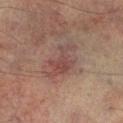Captured during whole-body skin photography for melanoma surveillance; the lesion was not biopsied. This image is a 15 mm lesion crop taken from a total-body photograph. This is a cross-polarized tile. Approximately 5 mm at its widest. The lesion is on the leg. A male patient aged 73 to 77.